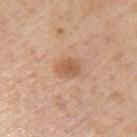This lesion was catalogued during total-body skin photography and was not selected for biopsy. The recorded lesion diameter is about 3 mm. A male subject roughly 75 years of age. Imaged with white-light lighting. Cropped from a whole-body photographic skin survey; the tile spans about 15 mm. On the left upper arm.